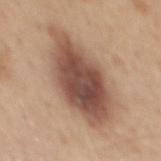This lesion was catalogued during total-body skin photography and was not selected for biopsy. Approximately 10 mm at its widest. A 15 mm close-up tile from a total-body photography series done for melanoma screening. The patient is a male aged approximately 45. Located on the mid back. The tile uses white-light illumination.